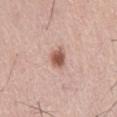Recorded during total-body skin imaging; not selected for excision or biopsy.
The subject is a male aged approximately 55.
A roughly 15 mm field-of-view crop from a total-body skin photograph.